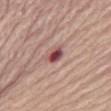Q: Was this lesion biopsied?
A: imaged on a skin check; not biopsied
Q: What is the anatomic site?
A: the chest
Q: How was the tile lit?
A: white-light
Q: What is the imaging modality?
A: 15 mm crop, total-body photography
Q: Automated lesion metrics?
A: a footprint of about 3.5 mm² and an eccentricity of roughly 0.8; a lesion–skin lightness drop of about 17 and a normalized border contrast of about 11.5
Q: What are the patient's age and sex?
A: female, aged approximately 65